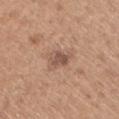Impression:
Captured during whole-body skin photography for melanoma surveillance; the lesion was not biopsied.